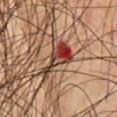biopsy_status: not biopsied; imaged during a skin examination
automated_metrics:
  eccentricity: 0.9
  shape_asymmetry: 0.6
  vs_skin_contrast_norm: 12.0
site: chest
image:
  source: total-body photography crop
  field_of_view_mm: 15
patient:
  sex: male
  age_approx: 65